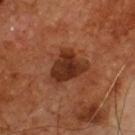Impression:
Part of a total-body skin-imaging series; this lesion was reviewed on a skin check and was not flagged for biopsy.
Clinical summary:
This is a cross-polarized tile. Located on the front of the torso. The recorded lesion diameter is about 5 mm. A 15 mm close-up tile from a total-body photography series done for melanoma screening. A male patient, aged approximately 55. Automated tile analysis of the lesion measured a shape eccentricity near 0.65 and a symmetry-axis asymmetry near 0.4. It also reported border irregularity of about 3.5 on a 0–10 scale, a within-lesion color-variation index near 4.5/10, and peripheral color asymmetry of about 1.5.